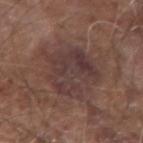acquisition: total-body-photography crop, ~15 mm field of view
TBP lesion metrics: a nevus-likeness score of about 0/100 and a detector confidence of about 90 out of 100 that the crop contains a lesion
location: the left forearm
subject: male, approximately 75 years of age
tile lighting: white-light